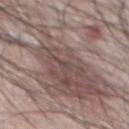{
  "biopsy_status": "not biopsied; imaged during a skin examination",
  "patient": {
    "sex": "male",
    "age_approx": 65
  },
  "site": "chest",
  "image": {
    "source": "total-body photography crop",
    "field_of_view_mm": 15
  }
}